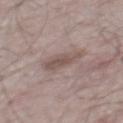| feature | finding |
|---|---|
| notes | total-body-photography surveillance lesion; no biopsy |
| illumination | white-light illumination |
| image | total-body-photography crop, ~15 mm field of view |
| lesion diameter | ≈4 mm |
| TBP lesion metrics | a normalized lesion–skin contrast near 7; a nevus-likeness score of about 5/100 and a lesion-detection confidence of about 100/100 |
| patient | male, aged around 65 |
| body site | the mid back |